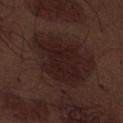anatomic site: the abdomen | illumination: white-light | lesion size: ≈6.5 mm | imaging modality: ~15 mm tile from a whole-body skin photo | automated lesion analysis: an area of roughly 18 mm², a shape eccentricity near 0.7, and a symmetry-axis asymmetry near 0.3; a lesion–skin lightness drop of about 6 and a normalized lesion–skin contrast near 8; a border-irregularity index near 4/10, a within-lesion color-variation index near 2.5/10, and radial color variation of about 1; a classifier nevus-likeness of about 0/100 and lesion-presence confidence of about 90/100 | subject: male, aged approximately 70.